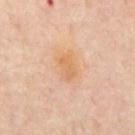No biopsy was performed on this lesion — it was imaged during a full skin examination and was not determined to be concerning. The patient is a male aged 63 to 67. On the mid back. Cropped from a whole-body photographic skin survey; the tile spans about 15 mm. The tile uses cross-polarized illumination.